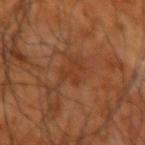<lesion>
  <biopsy_status>not biopsied; imaged during a skin examination</biopsy_status>
  <site>right upper arm</site>
  <lesion_size>
    <long_diameter_mm_approx>3.0</long_diameter_mm_approx>
  </lesion_size>
  <lighting>cross-polarized</lighting>
  <patient>
    <sex>male</sex>
    <age_approx>65</age_approx>
  </patient>
  <image>
    <source>total-body photography crop</source>
    <field_of_view_mm>15</field_of_view_mm>
  </image>
</lesion>